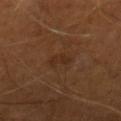Clinical impression:
This lesion was catalogued during total-body skin photography and was not selected for biopsy.
Acquisition and patient details:
A male subject, aged 63–67. A 15 mm close-up tile from a total-body photography series done for melanoma screening. Captured under cross-polarized illumination. The total-body-photography lesion software estimated an area of roughly 3 mm², an outline eccentricity of about 0.8 (0 = round, 1 = elongated), and a symmetry-axis asymmetry near 0.35. About 2.5 mm across.